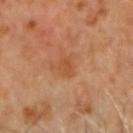* follow-up · total-body-photography surveillance lesion; no biopsy
* lighting · cross-polarized
* patient · male, approximately 60 years of age
* imaging modality · 15 mm crop, total-body photography
* lesion diameter · ~2.5 mm (longest diameter)
* anatomic site · the head or neck